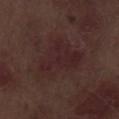  biopsy_status: not biopsied; imaged during a skin examination
  site: left thigh
  image:
    source: total-body photography crop
    field_of_view_mm: 15
  lesion_size:
    long_diameter_mm_approx: 6.5
  lighting: white-light
  automated_metrics:
    area_mm2_approx: 22.0
    eccentricity: 0.6
    shape_asymmetry: 0.4
    cielab_L: 24
    cielab_a: 18
    cielab_b: 15
    vs_skin_darker_L: 4.0
  patient:
    sex: male
    age_approx: 70